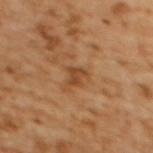Impression:
No biopsy was performed on this lesion — it was imaged during a full skin examination and was not determined to be concerning.
Background:
The lesion is on the upper back. Automated image analysis of the tile measured an outline eccentricity of about 0.75 (0 = round, 1 = elongated) and a symmetry-axis asymmetry near 0.4. The software also gave a border-irregularity index near 4/10, a within-lesion color-variation index near 1.5/10, and radial color variation of about 0.5. It also reported a detector confidence of about 100 out of 100 that the crop contains a lesion. A female patient aged 53 to 57. Approximately 2.5 mm at its widest. Cropped from a total-body skin-imaging series; the visible field is about 15 mm.